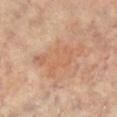Clinical impression: The lesion was photographed on a routine skin check and not biopsied; there is no pathology result. Image and clinical context: The lesion is on the left leg. This is a cross-polarized tile. Automated tile analysis of the lesion measured an outline eccentricity of about 0.9 (0 = round, 1 = elongated). The analysis additionally found a mean CIELAB color near L≈59 a*≈20 b*≈33. Cropped from a total-body skin-imaging series; the visible field is about 15 mm. A female patient aged approximately 65.